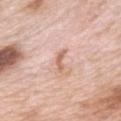notes — no biopsy performed (imaged during a skin exam) | body site — the upper back | image — ~15 mm crop, total-body skin-cancer survey | subject — female, roughly 65 years of age | automated metrics — an area of roughly 2.5 mm², a shape eccentricity near 0.9, and a shape-asymmetry score of about 0.6 (0 = symmetric); an average lesion color of about L≈65 a*≈22 b*≈30 (CIELAB), roughly 11 lightness units darker than nearby skin, and a normalized border contrast of about 7; border irregularity of about 6.5 on a 0–10 scale, a color-variation rating of about 0/10, and a peripheral color-asymmetry measure near 0; an automated nevus-likeness rating near 0 out of 100 and a detector confidence of about 100 out of 100 that the crop contains a lesion | size — ≈3 mm | tile lighting — white-light.